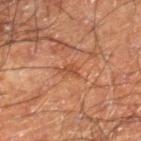Clinical impression: Captured during whole-body skin photography for melanoma surveillance; the lesion was not biopsied. Image and clinical context: The lesion is on the leg. A male subject roughly 60 years of age. A 15 mm crop from a total-body photograph taken for skin-cancer surveillance.